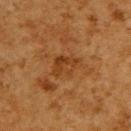Q: Where on the body is the lesion?
A: the upper back
Q: Who is the patient?
A: male, approximately 60 years of age
Q: What did automated image analysis measure?
A: a footprint of about 7 mm², an outline eccentricity of about 0.7 (0 = round, 1 = elongated), and a symmetry-axis asymmetry near 0.35; border irregularity of about 4 on a 0–10 scale and a color-variation rating of about 4/10; lesion-presence confidence of about 100/100
Q: What lighting was used for the tile?
A: cross-polarized
Q: How was this image acquired?
A: ~15 mm crop, total-body skin-cancer survey
Q: How large is the lesion?
A: ~3.5 mm (longest diameter)